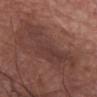follow-up: imaged on a skin check; not biopsied | subject: female, roughly 75 years of age | imaging modality: ~15 mm tile from a whole-body skin photo | location: the left thigh | automated metrics: a footprint of about 20 mm², an outline eccentricity of about 0.9 (0 = round, 1 = elongated), and two-axis asymmetry of about 0.5; a border-irregularity rating of about 7.5/10.